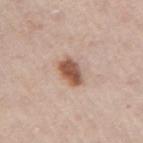Findings:
• follow-up — no biopsy performed (imaged during a skin exam)
• body site — the right upper arm
• diameter — ≈3.5 mm
• subject — female, aged around 50
• TBP lesion metrics — a mean CIELAB color near L≈54 a*≈21 b*≈29, roughly 16 lightness units darker than nearby skin, and a normalized border contrast of about 11; a within-lesion color-variation index near 4/10 and peripheral color asymmetry of about 1.5; a classifier nevus-likeness of about 100/100 and a lesion-detection confidence of about 100/100
• image — ~15 mm tile from a whole-body skin photo